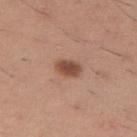Impression: Imaged during a routine full-body skin examination; the lesion was not biopsied and no histopathology is available. Acquisition and patient details: Located on the right upper arm. The lesion's longest dimension is about 3 mm. A male subject, roughly 35 years of age. The total-body-photography lesion software estimated border irregularity of about 1.5 on a 0–10 scale, internal color variation of about 3 on a 0–10 scale, and a peripheral color-asymmetry measure near 1. The tile uses white-light illumination. A roughly 15 mm field-of-view crop from a total-body skin photograph.